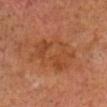{
  "biopsy_status": "not biopsied; imaged during a skin examination",
  "patient": {
    "sex": "male",
    "age_approx": 70
  },
  "lesion_size": {
    "long_diameter_mm_approx": 6.0
  },
  "lighting": "cross-polarized",
  "image": {
    "source": "total-body photography crop",
    "field_of_view_mm": 15
  },
  "site": "head or neck"
}